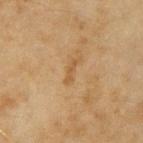Q: Is there a histopathology result?
A: catalogued during a skin exam; not biopsied
Q: Lesion location?
A: the right forearm
Q: Patient demographics?
A: female, roughly 55 years of age
Q: What kind of image is this?
A: ~15 mm crop, total-body skin-cancer survey